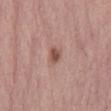Findings:
- patient: male, in their mid-60s
- body site: the mid back
- imaging modality: ~15 mm tile from a whole-body skin photo
- tile lighting: white-light
- lesion diameter: ≈2.5 mm